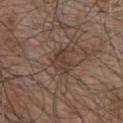Impression: No biopsy was performed on this lesion — it was imaged during a full skin examination and was not determined to be concerning. Context: The subject is a male in their mid- to late 50s. Captured under white-light illumination. From the upper back. This image is a 15 mm lesion crop taken from a total-body photograph. Measured at roughly 3 mm in maximum diameter.